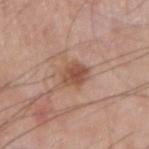Case summary:
- workup — catalogued during a skin exam; not biopsied
- lighting — white-light
- patient — male, approximately 65 years of age
- body site — the right upper arm
- imaging modality — ~15 mm tile from a whole-body skin photo